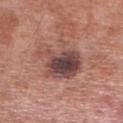follow-up = no biopsy performed (imaged during a skin exam)
location = the right lower leg
diameter = ~6 mm (longest diameter)
tile lighting = white-light
image source = ~15 mm crop, total-body skin-cancer survey
subject = male, in their mid- to late 50s